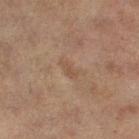Findings:
- notes · total-body-photography surveillance lesion; no biopsy
- automated metrics · an average lesion color of about L≈44 a*≈16 b*≈26 (CIELAB), about 5 CIELAB-L* units darker than the surrounding skin, and a lesion-to-skin contrast of about 5 (normalized; higher = more distinct); a border-irregularity rating of about 4/10 and peripheral color asymmetry of about 0
- body site · the left thigh
- diameter · ≈2.5 mm
- patient · female, roughly 60 years of age
- illumination · cross-polarized
- image · 15 mm crop, total-body photography